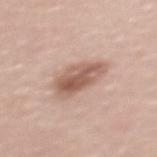This lesion was catalogued during total-body skin photography and was not selected for biopsy. A female subject, aged approximately 40. The lesion's longest dimension is about 5 mm. Imaged with white-light lighting. Automated tile analysis of the lesion measured lesion-presence confidence of about 100/100. The lesion is located on the upper back. A region of skin cropped from a whole-body photographic capture, roughly 15 mm wide.